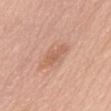The lesion was photographed on a routine skin check and not biopsied; there is no pathology result. Captured under white-light illumination. A 15 mm crop from a total-body photograph taken for skin-cancer surveillance. The patient is a female aged approximately 60. About 3.5 mm across. The lesion is on the mid back.